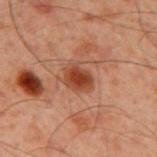| field | value |
|---|---|
| biopsy status | no biopsy performed (imaged during a skin exam) |
| diameter | ≈3.5 mm |
| imaging modality | 15 mm crop, total-body photography |
| subject | male, in their 60s |
| lighting | cross-polarized |
| anatomic site | the mid back |